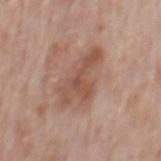Recorded during total-body skin imaging; not selected for excision or biopsy.
Measured at roughly 6 mm in maximum diameter.
This is a white-light tile.
A region of skin cropped from a whole-body photographic capture, roughly 15 mm wide.
The lesion is on the back.
The patient is a male roughly 70 years of age.